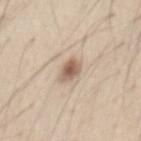Q: Was this lesion biopsied?
A: imaged on a skin check; not biopsied
Q: What is the anatomic site?
A: the abdomen
Q: What are the patient's age and sex?
A: male, about 45 years old
Q: What kind of image is this?
A: ~15 mm tile from a whole-body skin photo